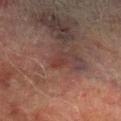Assessment:
No biopsy was performed on this lesion — it was imaged during a full skin examination and was not determined to be concerning.
Acquisition and patient details:
A male subject, aged 73–77. Captured under cross-polarized illumination. From the right lower leg. A region of skin cropped from a whole-body photographic capture, roughly 15 mm wide. Approximately 2.5 mm at its widest.